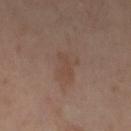Part of a total-body skin-imaging series; this lesion was reviewed on a skin check and was not flagged for biopsy.
A female subject, roughly 50 years of age.
About 3 mm across.
The tile uses cross-polarized illumination.
A region of skin cropped from a whole-body photographic capture, roughly 15 mm wide.
The lesion is located on the left thigh.